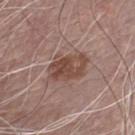patient: male, aged approximately 70 | image: total-body-photography crop, ~15 mm field of view | lighting: white-light illumination | lesion diameter: ≈5 mm | automated lesion analysis: a footprint of about 13 mm², an outline eccentricity of about 0.75 (0 = round, 1 = elongated), and a shape-asymmetry score of about 0.2 (0 = symmetric) | location: the front of the torso.